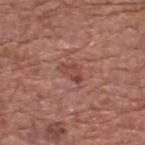notes: no biopsy performed (imaged during a skin exam); body site: the upper back; tile lighting: white-light illumination; subject: male, aged approximately 75; lesion size: about 3 mm; acquisition: total-body-photography crop, ~15 mm field of view.